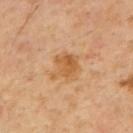Automated tile analysis of the lesion measured an automated nevus-likeness rating near 20 out of 100.
A male patient, aged 53–57.
A lesion tile, about 15 mm wide, cut from a 3D total-body photograph.
The lesion's longest dimension is about 3.5 mm.
Imaged with cross-polarized lighting.
Located on the mid back.